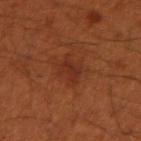The lesion was tiled from a total-body skin photograph and was not biopsied. A 15 mm crop from a total-body photograph taken for skin-cancer surveillance. A male patient in their mid-50s. From the arm.